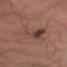The lesion was photographed on a routine skin check and not biopsied; there is no pathology result. Measured at roughly 8.5 mm in maximum diameter. A male subject roughly 45 years of age. The lesion-visualizer software estimated a mean CIELAB color near L≈45 a*≈18 b*≈23, about 7 CIELAB-L* units darker than the surrounding skin, and a normalized lesion–skin contrast near 5.5. It also reported a classifier nevus-likeness of about 20/100 and a lesion-detection confidence of about 100/100. A region of skin cropped from a whole-body photographic capture, roughly 15 mm wide. This is a white-light tile. On the left upper arm.